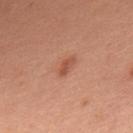{"biopsy_status": "not biopsied; imaged during a skin examination", "site": "upper back", "patient": {"sex": "male", "age_approx": 70}, "image": {"source": "total-body photography crop", "field_of_view_mm": 15}, "lighting": "white-light", "lesion_size": {"long_diameter_mm_approx": 2.5}}